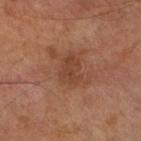<case>
<biopsy_status>not biopsied; imaged during a skin examination</biopsy_status>
<lesion_size>
  <long_diameter_mm_approx>3.5</long_diameter_mm_approx>
</lesion_size>
<automated_metrics>
  <area_mm2_approx>6.5</area_mm2_approx>
  <eccentricity>0.7</eccentricity>
  <shape_asymmetry>0.3</shape_asymmetry>
  <border_irregularity_0_10>3.5</border_irregularity_0_10>
  <color_variation_0_10>2.5</color_variation_0_10>
  <peripheral_color_asymmetry>1.0</peripheral_color_asymmetry>
</automated_metrics>
<site>right lower leg</site>
<image>
  <source>total-body photography crop</source>
  <field_of_view_mm>15</field_of_view_mm>
</image>
<patient>
  <sex>male</sex>
  <age_approx>70</age_approx>
</patient>
</case>